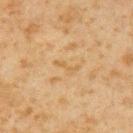| key | value |
|---|---|
| biopsy status | total-body-photography surveillance lesion; no biopsy |
| patient | male, aged 58–62 |
| location | the left upper arm |
| imaging modality | ~15 mm crop, total-body skin-cancer survey |
| size | ≈3 mm |
| tile lighting | cross-polarized illumination |